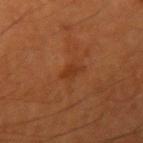workup = imaged on a skin check; not biopsied | lighting = cross-polarized illumination | image = 15 mm crop, total-body photography | lesion diameter = ≈2.5 mm | anatomic site = the right upper arm | image-analysis metrics = an area of roughly 2.5 mm², an eccentricity of roughly 0.9, and a symmetry-axis asymmetry near 0.35; a lesion color around L≈32 a*≈24 b*≈33 in CIELAB and a lesion–skin lightness drop of about 6 | patient = male, aged around 55.